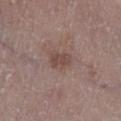Q: Was this lesion biopsied?
A: total-body-photography surveillance lesion; no biopsy
Q: What kind of image is this?
A: total-body-photography crop, ~15 mm field of view
Q: Automated lesion metrics?
A: an automated nevus-likeness rating near 5 out of 100
Q: Who is the patient?
A: female, in their mid-80s or older
Q: Lesion size?
A: ~3 mm (longest diameter)
Q: Where on the body is the lesion?
A: the left lower leg